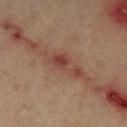biopsy status — catalogued during a skin exam; not biopsied | anatomic site — the left lower leg | acquisition — ~15 mm crop, total-body skin-cancer survey | subject — aged approximately 60.